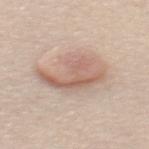Clinical impression: Recorded during total-body skin imaging; not selected for excision or biopsy. Acquisition and patient details: Cropped from a total-body skin-imaging series; the visible field is about 15 mm. The patient is a female aged 53 to 57. Located on the upper back. This is a white-light tile. Approximately 6.5 mm at its widest.